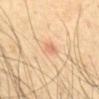A male patient aged approximately 45. Located on the front of the torso. A 15 mm close-up extracted from a 3D total-body photography capture.